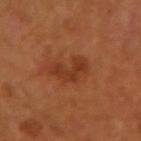workup — no biopsy performed (imaged during a skin exam); image — ~15 mm tile from a whole-body skin photo; illumination — cross-polarized; diameter — ≈5 mm; site — the arm; subject — female, in their mid- to late 50s.